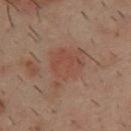Impression:
Recorded during total-body skin imaging; not selected for excision or biopsy.
Image and clinical context:
A close-up tile cropped from a whole-body skin photograph, about 15 mm across. A male subject, aged 38–42. Captured under cross-polarized illumination. Located on the upper back.